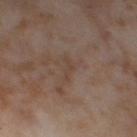Part of a total-body skin-imaging series; this lesion was reviewed on a skin check and was not flagged for biopsy.
The lesion-visualizer software estimated a lesion area of about 2 mm², an eccentricity of roughly 0.9, and a shape-asymmetry score of about 0.55 (0 = symmetric). The analysis additionally found a lesion color around L≈42 a*≈15 b*≈25 in CIELAB and a normalized lesion–skin contrast near 5. And it measured internal color variation of about 0 on a 0–10 scale and peripheral color asymmetry of about 0. The software also gave a lesion-detection confidence of about 95/100.
Imaged with cross-polarized lighting.
The lesion's longest dimension is about 2.5 mm.
The lesion is located on the right thigh.
A close-up tile cropped from a whole-body skin photograph, about 15 mm across.
The subject is a female in their mid- to late 50s.